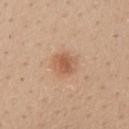Clinical impression: Part of a total-body skin-imaging series; this lesion was reviewed on a skin check and was not flagged for biopsy. Context: The patient is a male in their 30s. The lesion's longest dimension is about 2.5 mm. This is a white-light tile. A roughly 15 mm field-of-view crop from a total-body skin photograph. Located on the mid back. Automated image analysis of the tile measured a within-lesion color-variation index near 2.5/10 and radial color variation of about 1.